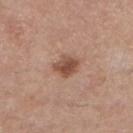Clinical impression:
The lesion was photographed on a routine skin check and not biopsied; there is no pathology result.
Clinical summary:
A female patient, aged 43–47. A close-up tile cropped from a whole-body skin photograph, about 15 mm across. The lesion is on the left lower leg. Automated image analysis of the tile measured a symmetry-axis asymmetry near 0.25. The analysis additionally found border irregularity of about 2.5 on a 0–10 scale, a color-variation rating of about 3/10, and peripheral color asymmetry of about 1. The recorded lesion diameter is about 3 mm.